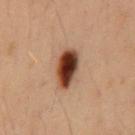Impression:
This lesion was catalogued during total-body skin photography and was not selected for biopsy.
Acquisition and patient details:
The subject is a male about 40 years old. The total-body-photography lesion software estimated an area of roughly 10 mm², an eccentricity of roughly 0.85, and a shape-asymmetry score of about 0.15 (0 = symmetric). And it measured a border-irregularity index near 2/10 and internal color variation of about 9.5 on a 0–10 scale. It also reported a detector confidence of about 100 out of 100 that the crop contains a lesion. A roughly 15 mm field-of-view crop from a total-body skin photograph. The lesion's longest dimension is about 5 mm. The tile uses cross-polarized illumination. The lesion is located on the mid back.